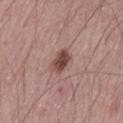Imaged during a routine full-body skin examination; the lesion was not biopsied and no histopathology is available. From the left thigh. About 3 mm across. A lesion tile, about 15 mm wide, cut from a 3D total-body photograph. This is a white-light tile. A male subject in their mid- to late 60s.